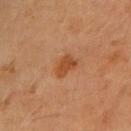notes = total-body-photography surveillance lesion; no biopsy
imaging modality = ~15 mm tile from a whole-body skin photo
subject = female, approximately 70 years of age
illumination = cross-polarized illumination
lesion diameter = ≈3 mm
body site = the left upper arm